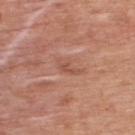biopsy status = total-body-photography surveillance lesion; no biopsy | lighting = white-light | lesion diameter = about 3.5 mm | location = the upper back | image source = total-body-photography crop, ~15 mm field of view | patient = male, aged around 70.